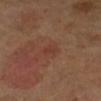<tbp_lesion>
<biopsy_status>not biopsied; imaged during a skin examination</biopsy_status>
<patient>
  <sex>female</sex>
  <age_approx>55</age_approx>
</patient>
<image>
  <source>total-body photography crop</source>
  <field_of_view_mm>15</field_of_view_mm>
</image>
<site>right lower leg</site>
</tbp_lesion>